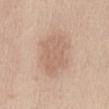Notes:
- notes · total-body-photography surveillance lesion; no biopsy
- acquisition · total-body-photography crop, ~15 mm field of view
- diameter · ~5 mm (longest diameter)
- patient · female, in their mid-40s
- location · the abdomen
- automated lesion analysis · a lesion area of about 17 mm² and two-axis asymmetry of about 0.15; a lesion color around L≈63 a*≈18 b*≈28 in CIELAB, about 8 CIELAB-L* units darker than the surrounding skin, and a lesion-to-skin contrast of about 5 (normalized; higher = more distinct); a border-irregularity index near 2.5/10 and a color-variation rating of about 2.5/10